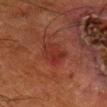Case summary:
– lesion diameter: ≈3.5 mm
– automated lesion analysis: about 5 CIELAB-L* units darker than the surrounding skin and a normalized border contrast of about 5.5; a border-irregularity rating of about 2.5/10 and internal color variation of about 4 on a 0–10 scale
– patient: male, in their 80s
– illumination: cross-polarized illumination
– anatomic site: the right lower leg
– imaging modality: total-body-photography crop, ~15 mm field of view